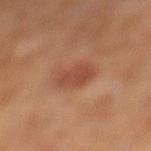Recorded during total-body skin imaging; not selected for excision or biopsy. On the left lower leg. Longest diameter approximately 4 mm. Cropped from a whole-body photographic skin survey; the tile spans about 15 mm. Imaged with cross-polarized lighting. Automated image analysis of the tile measured a footprint of about 7 mm², an eccentricity of roughly 0.85, and a symmetry-axis asymmetry near 0.25. The software also gave a lesion-detection confidence of about 100/100. The subject is a female in their 60s.